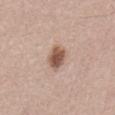Recorded during total-body skin imaging; not selected for excision or biopsy. The tile uses white-light illumination. Longest diameter approximately 3 mm. From the lower back. Automated tile analysis of the lesion measured a footprint of about 6 mm², a shape eccentricity near 0.6, and two-axis asymmetry of about 0.2. The analysis additionally found a mean CIELAB color near L≈54 a*≈19 b*≈27 and a lesion-to-skin contrast of about 10 (normalized; higher = more distinct). And it measured an automated nevus-likeness rating near 95 out of 100 and a lesion-detection confidence of about 100/100. A lesion tile, about 15 mm wide, cut from a 3D total-body photograph. A male subject, aged approximately 45.